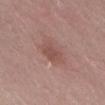No biopsy was performed on this lesion — it was imaged during a full skin examination and was not determined to be concerning.
The recorded lesion diameter is about 3.5 mm.
Cropped from a total-body skin-imaging series; the visible field is about 15 mm.
The lesion-visualizer software estimated a footprint of about 6.5 mm² and an eccentricity of roughly 0.8. It also reported a border-irregularity rating of about 2/10, a within-lesion color-variation index near 2/10, and peripheral color asymmetry of about 0.5. The software also gave an automated nevus-likeness rating near 5 out of 100.
A female patient aged around 50.
On the abdomen.
Imaged with white-light lighting.